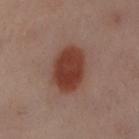The lesion was tiled from a total-body skin photograph and was not biopsied. The lesion is on the right leg. Captured under cross-polarized illumination. A close-up tile cropped from a whole-body skin photograph, about 15 mm across. A female subject, aged around 60. Longest diameter approximately 5.5 mm.